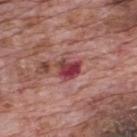Clinical impression:
No biopsy was performed on this lesion — it was imaged during a full skin examination and was not determined to be concerning.
Acquisition and patient details:
A male subject, aged 68–72. The tile uses white-light illumination. A roughly 15 mm field-of-view crop from a total-body skin photograph. Located on the upper back. About 3 mm across.